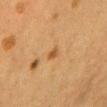| feature | finding |
|---|---|
| biopsy status | no biopsy performed (imaged during a skin exam) |
| patient | female, about 40 years old |
| body site | the chest |
| image source | 15 mm crop, total-body photography |
| image-analysis metrics | a footprint of about 4 mm², an eccentricity of roughly 0.65, and a shape-asymmetry score of about 0.25 (0 = symmetric); roughly 6 lightness units darker than nearby skin and a lesion-to-skin contrast of about 5 (normalized; higher = more distinct); an automated nevus-likeness rating near 25 out of 100 |